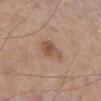No biopsy was performed on this lesion — it was imaged during a full skin examination and was not determined to be concerning. On the left lower leg. A roughly 15 mm field-of-view crop from a total-body skin photograph. The lesion-visualizer software estimated a footprint of about 7 mm² and an outline eccentricity of about 0.7 (0 = round, 1 = elongated). The software also gave a border-irregularity index near 3/10, a color-variation rating of about 4.5/10, and a peripheral color-asymmetry measure near 1.5. It also reported a nevus-likeness score of about 55/100 and lesion-presence confidence of about 100/100. A male patient in their mid- to late 50s.